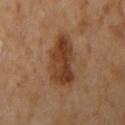Image and clinical context:
Automated image analysis of the tile measured a footprint of about 16 mm², an outline eccentricity of about 0.8 (0 = round, 1 = elongated), and a symmetry-axis asymmetry near 0.25. It also reported a border-irregularity rating of about 3/10, internal color variation of about 5 on a 0–10 scale, and peripheral color asymmetry of about 2. A female subject aged 58 to 62. The tile uses cross-polarized illumination. A 15 mm close-up tile from a total-body photography series done for melanoma screening. Measured at roughly 5.5 mm in maximum diameter. From the right upper arm.